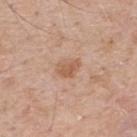biopsy status = no biopsy performed (imaged during a skin exam)
acquisition = ~15 mm tile from a whole-body skin photo
diameter = ≈3 mm
body site = the upper back
subject = male, in their 60s
tile lighting = white-light illumination